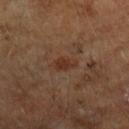Longest diameter approximately 3 mm.
The patient is a male roughly 65 years of age.
A region of skin cropped from a whole-body photographic capture, roughly 15 mm wide.
Captured under cross-polarized illumination.
From the arm.